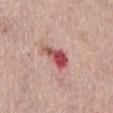Recorded during total-body skin imaging; not selected for excision or biopsy.
A female subject aged 63–67.
A roughly 15 mm field-of-view crop from a total-body skin photograph.
The lesion is located on the abdomen.
Measured at roughly 4 mm in maximum diameter.
Captured under white-light illumination.
The lesion-visualizer software estimated an outline eccentricity of about 0.85 (0 = round, 1 = elongated). And it measured a mean CIELAB color near L≈52 a*≈32 b*≈24, a lesion–skin lightness drop of about 16, and a normalized lesion–skin contrast near 10.5. It also reported border irregularity of about 4.5 on a 0–10 scale, a within-lesion color-variation index near 9/10, and peripheral color asymmetry of about 4. The software also gave a nevus-likeness score of about 0/100 and a detector confidence of about 100 out of 100 that the crop contains a lesion.